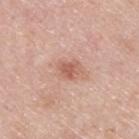{
  "biopsy_status": "not biopsied; imaged during a skin examination",
  "patient": {
    "sex": "male",
    "age_approx": 45
  },
  "image": {
    "source": "total-body photography crop",
    "field_of_view_mm": 15
  },
  "site": "back",
  "lighting": "white-light"
}